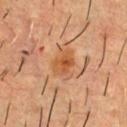Clinical impression: The lesion was photographed on a routine skin check and not biopsied; there is no pathology result. Image and clinical context: The lesion-visualizer software estimated an area of roughly 6 mm², a shape eccentricity near 0.7, and a symmetry-axis asymmetry near 0.25. And it measured border irregularity of about 2.5 on a 0–10 scale, internal color variation of about 4 on a 0–10 scale, and a peripheral color-asymmetry measure near 1.5. The analysis additionally found a classifier nevus-likeness of about 65/100 and lesion-presence confidence of about 100/100. This image is a 15 mm lesion crop taken from a total-body photograph. The tile uses cross-polarized illumination. On the upper back. The subject is a male aged around 55.